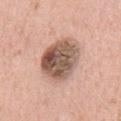The lesion was photographed on a routine skin check and not biopsied; there is no pathology result.
Approximately 5.5 mm at its widest.
The tile uses white-light illumination.
Located on the chest.
The subject is a male in their mid-50s.
A 15 mm crop from a total-body photograph taken for skin-cancer surveillance.